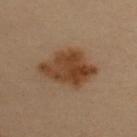notes: catalogued during a skin exam; not biopsied | lighting: cross-polarized | subject: female, aged 48 to 52 | image-analysis metrics: a mean CIELAB color near L≈35 a*≈16 b*≈28, roughly 10 lightness units darker than nearby skin, and a normalized border contrast of about 10; border irregularity of about 2.5 on a 0–10 scale, a color-variation rating of about 4/10, and radial color variation of about 1.5; a nevus-likeness score of about 90/100 | acquisition: total-body-photography crop, ~15 mm field of view | lesion diameter: about 6.5 mm | body site: the chest.